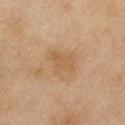Clinical impression: Recorded during total-body skin imaging; not selected for excision or biopsy. Background: A region of skin cropped from a whole-body photographic capture, roughly 15 mm wide. A female subject aged approximately 60. The lesion is on the left thigh. Imaged with cross-polarized lighting. Longest diameter approximately 3.5 mm.